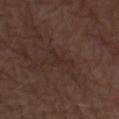Q: Was this lesion biopsied?
A: catalogued during a skin exam; not biopsied
Q: Automated lesion metrics?
A: a footprint of about 3 mm² and a shape eccentricity near 0.9; an automated nevus-likeness rating near 0 out of 100 and a lesion-detection confidence of about 80/100
Q: What kind of image is this?
A: ~15 mm crop, total-body skin-cancer survey
Q: Where on the body is the lesion?
A: the leg
Q: How was the tile lit?
A: white-light
Q: What are the patient's age and sex?
A: male, in their 70s